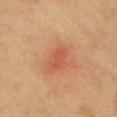<case>
  <biopsy_status>not biopsied; imaged during a skin examination</biopsy_status>
</case>